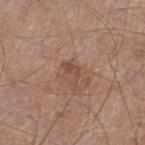Q: Is there a histopathology result?
A: catalogued during a skin exam; not biopsied
Q: What lighting was used for the tile?
A: white-light illumination
Q: What are the patient's age and sex?
A: male, about 60 years old
Q: Where on the body is the lesion?
A: the left lower leg
Q: Automated lesion metrics?
A: border irregularity of about 6.5 on a 0–10 scale and radial color variation of about 0; lesion-presence confidence of about 100/100
Q: What kind of image is this?
A: ~15 mm crop, total-body skin-cancer survey
Q: Lesion size?
A: ≈3 mm